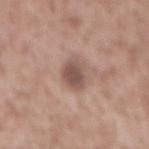| key | value |
|---|---|
| notes | total-body-photography surveillance lesion; no biopsy |
| image-analysis metrics | a lesion area of about 7 mm² and a shape eccentricity near 0.8; an average lesion color of about L≈52 a*≈18 b*≈23 (CIELAB), about 11 CIELAB-L* units darker than the surrounding skin, and a normalized lesion–skin contrast near 8 |
| illumination | white-light illumination |
| location | the mid back |
| lesion size | about 4 mm |
| subject | male, aged around 45 |
| imaging modality | ~15 mm crop, total-body skin-cancer survey |